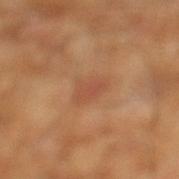Findings:
• workup · imaged on a skin check; not biopsied
• imaging modality · total-body-photography crop, ~15 mm field of view
• tile lighting · cross-polarized
• size · ~3 mm (longest diameter)
• TBP lesion metrics · a border-irregularity index near 3.5/10, a color-variation rating of about 2/10, and a peripheral color-asymmetry measure near 0.5
• patient · male, about 65 years old